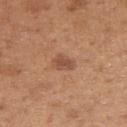notes: catalogued during a skin exam; not biopsied
image-analysis metrics: a border-irregularity index near 2.5/10 and a peripheral color-asymmetry measure near 1
acquisition: total-body-photography crop, ~15 mm field of view
lighting: white-light illumination
site: the left upper arm
patient: female, about 30 years old